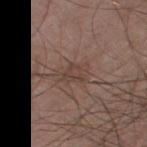Impression: The lesion was tiled from a total-body skin photograph and was not biopsied. Image and clinical context: The recorded lesion diameter is about 3.5 mm. A 15 mm close-up tile from a total-body photography series done for melanoma screening. The lesion is on the left thigh. Captured under white-light illumination. A male patient, in their 60s. Automated image analysis of the tile measured a lesion color around L≈42 a*≈16 b*≈23 in CIELAB, roughly 6 lightness units darker than nearby skin, and a normalized border contrast of about 5.5.